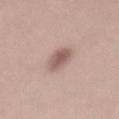follow-up: imaged on a skin check; not biopsied
diameter: about 3.5 mm
automated lesion analysis: an area of roughly 6.5 mm², a shape eccentricity near 0.8, and two-axis asymmetry of about 0.2; a color-variation rating of about 3/10 and a peripheral color-asymmetry measure near 1
site: the mid back
image: ~15 mm tile from a whole-body skin photo
subject: female, aged around 25
illumination: white-light